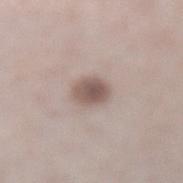<tbp_lesion>
  <biopsy_status>not biopsied; imaged during a skin examination</biopsy_status>
  <image>
    <source>total-body photography crop</source>
    <field_of_view_mm>15</field_of_view_mm>
  </image>
  <lesion_size>
    <long_diameter_mm_approx>3.0</long_diameter_mm_approx>
  </lesion_size>
  <site>left lower leg</site>
  <patient>
    <sex>male</sex>
    <age_approx>65</age_approx>
  </patient>
  <lighting>white-light</lighting>
</tbp_lesion>